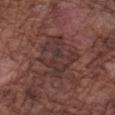The lesion's longest dimension is about 5.5 mm.
A 15 mm crop from a total-body photograph taken for skin-cancer surveillance.
The tile uses white-light illumination.
The lesion-visualizer software estimated a shape eccentricity near 0.65 and two-axis asymmetry of about 0.35. And it measured a lesion–skin lightness drop of about 6 and a normalized border contrast of about 6.5. The software also gave internal color variation of about 4 on a 0–10 scale.
Located on the left forearm.
The subject is a male about 75 years old.